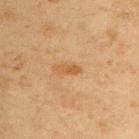notes — imaged on a skin check; not biopsied.